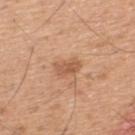The lesion is located on the upper back. Automated image analysis of the tile measured a footprint of about 4.5 mm² and an eccentricity of roughly 0.85. Captured under white-light illumination. Cropped from a total-body skin-imaging series; the visible field is about 15 mm. A male subject roughly 45 years of age. The lesion's longest dimension is about 3 mm.